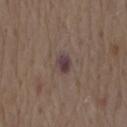follow-up: no biopsy performed (imaged during a skin exam); lighting: white-light; body site: the back; diameter: ≈2.5 mm; patient: male, roughly 70 years of age; imaging modality: total-body-photography crop, ~15 mm field of view.